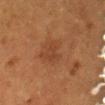- patient — female, aged 48–52
- body site — the lower back
- image source — ~15 mm crop, total-body skin-cancer survey